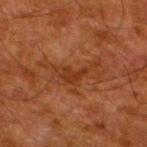notes=catalogued during a skin exam; not biopsied
acquisition=~15 mm crop, total-body skin-cancer survey
tile lighting=cross-polarized illumination
anatomic site=the left lower leg
lesion size=~6.5 mm (longest diameter)
patient=male, aged approximately 80
automated metrics=a mean CIELAB color near L≈29 a*≈21 b*≈29, about 5 CIELAB-L* units darker than the surrounding skin, and a normalized border contrast of about 5.5; a border-irregularity rating of about 8.5/10, a within-lesion color-variation index near 3.5/10, and radial color variation of about 1; an automated nevus-likeness rating near 0 out of 100 and a detector confidence of about 60 out of 100 that the crop contains a lesion